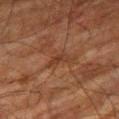Assessment: No biopsy was performed on this lesion — it was imaged during a full skin examination and was not determined to be concerning. Background: From the right thigh. A male subject, roughly 80 years of age. Automated image analysis of the tile measured two-axis asymmetry of about 0.5. And it measured a lesion color around L≈29 a*≈18 b*≈25 in CIELAB and about 5 CIELAB-L* units darker than the surrounding skin. And it measured border irregularity of about 5.5 on a 0–10 scale, a within-lesion color-variation index near 0/10, and a peripheral color-asymmetry measure near 0. This image is a 15 mm lesion crop taken from a total-body photograph. Approximately 3.5 mm at its widest. Captured under cross-polarized illumination.